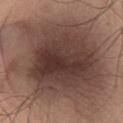notes = imaged on a skin check; not biopsied
lesion size = about 13 mm
imaging modality = ~15 mm tile from a whole-body skin photo
site = the abdomen
patient = male, in their mid-60s
lighting = white-light